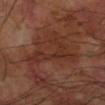Context:
About 7 mm across. The subject is a male aged around 70. The tile uses cross-polarized illumination. A 15 mm close-up extracted from a 3D total-body photography capture. The lesion is located on the left forearm.